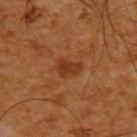This is a cross-polarized tile.
About 3 mm across.
This image is a 15 mm lesion crop taken from a total-body photograph.
A male patient, roughly 65 years of age.
On the upper back.